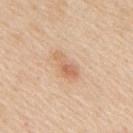From the mid back. A male patient aged 58–62. A region of skin cropped from a whole-body photographic capture, roughly 15 mm wide. Imaged with white-light lighting.